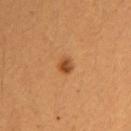{
  "biopsy_status": "not biopsied; imaged during a skin examination",
  "automated_metrics": {
    "eccentricity": 0.5,
    "shape_asymmetry": 0.15,
    "cielab_L": 48,
    "cielab_a": 26,
    "cielab_b": 41,
    "vs_skin_darker_L": 12.0,
    "vs_skin_contrast_norm": 8.5,
    "peripheral_color_asymmetry": 1.5,
    "lesion_detection_confidence_0_100": 100
  },
  "lesion_size": {
    "long_diameter_mm_approx": 2.0
  },
  "patient": {
    "sex": "female",
    "age_approx": 40
  },
  "image": {
    "source": "total-body photography crop",
    "field_of_view_mm": 15
  },
  "site": "arm",
  "lighting": "cross-polarized"
}